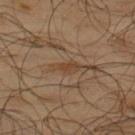A close-up tile cropped from a whole-body skin photograph, about 15 mm across.
The tile uses cross-polarized illumination.
A male subject aged approximately 65.
The lesion is located on the upper back.